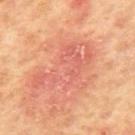| feature | finding |
|---|---|
| notes | catalogued during a skin exam; not biopsied |
| anatomic site | the mid back |
| TBP lesion metrics | a footprint of about 23 mm², a shape eccentricity near 0.85, and a symmetry-axis asymmetry near 0.5; a lesion color around L≈56 a*≈28 b*≈30 in CIELAB, roughly 6 lightness units darker than nearby skin, and a normalized border contrast of about 5; border irregularity of about 7 on a 0–10 scale, a within-lesion color-variation index near 4.5/10, and radial color variation of about 1.5; a classifier nevus-likeness of about 0/100 |
| illumination | cross-polarized |
| subject | male, aged around 65 |
| imaging modality | total-body-photography crop, ~15 mm field of view |
| lesion size | ≈8 mm |